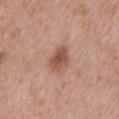Q: Is there a histopathology result?
A: no biopsy performed (imaged during a skin exam)
Q: How was the tile lit?
A: white-light
Q: Who is the patient?
A: male, roughly 50 years of age
Q: How was this image acquired?
A: ~15 mm crop, total-body skin-cancer survey
Q: What is the anatomic site?
A: the left upper arm
Q: What is the lesion's diameter?
A: about 3.5 mm